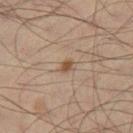Background: The lesion-visualizer software estimated an eccentricity of roughly 0.7 and a shape-asymmetry score of about 0.25 (0 = symmetric). It also reported a nevus-likeness score of about 95/100. A 15 mm close-up tile from a total-body photography series done for melanoma screening. The lesion is located on the right thigh. Captured under cross-polarized illumination. The recorded lesion diameter is about 1.5 mm. The subject is a male in their mid- to late 40s.